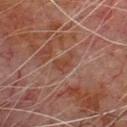A region of skin cropped from a whole-body photographic capture, roughly 15 mm wide.
Measured at roughly 3 mm in maximum diameter.
The tile uses cross-polarized illumination.
From the chest.
The total-body-photography lesion software estimated a footprint of about 3 mm² and a shape-asymmetry score of about 0.45 (0 = symmetric). It also reported a mean CIELAB color near L≈32 a*≈19 b*≈23, a lesion–skin lightness drop of about 6, and a normalized border contrast of about 6.5. And it measured border irregularity of about 4.5 on a 0–10 scale and a within-lesion color-variation index near 1/10. And it measured a nevus-likeness score of about 0/100 and lesion-presence confidence of about 80/100.
The subject is a male approximately 80 years of age.